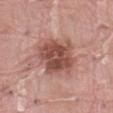Impression: The lesion was photographed on a routine skin check and not biopsied; there is no pathology result. Image and clinical context: The subject is a male aged 38 to 42. The lesion is located on the front of the torso. The recorded lesion diameter is about 5 mm. Automated image analysis of the tile measured a footprint of about 19 mm², an outline eccentricity of about 0.35 (0 = round, 1 = elongated), and two-axis asymmetry of about 0.2. The software also gave a classifier nevus-likeness of about 25/100 and a lesion-detection confidence of about 100/100. A roughly 15 mm field-of-view crop from a total-body skin photograph.